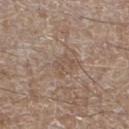Q: Was a biopsy performed?
A: imaged on a skin check; not biopsied
Q: Lesion size?
A: ≈2.5 mm
Q: How was this image acquired?
A: ~15 mm tile from a whole-body skin photo
Q: What are the patient's age and sex?
A: male, in their mid- to late 60s
Q: Lesion location?
A: the left lower leg
Q: What lighting was used for the tile?
A: white-light